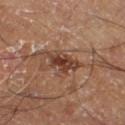Q: Was a biopsy performed?
A: catalogued during a skin exam; not biopsied
Q: Lesion location?
A: the right thigh
Q: What is the lesion's diameter?
A: ≈5 mm
Q: How was this image acquired?
A: 15 mm crop, total-body photography
Q: Illumination type?
A: cross-polarized illumination
Q: Patient demographics?
A: male, aged around 60
Q: Automated lesion metrics?
A: an area of roughly 7 mm², an eccentricity of roughly 0.9, and two-axis asymmetry of about 0.35; a within-lesion color-variation index near 3.5/10 and a peripheral color-asymmetry measure near 1; an automated nevus-likeness rating near 45 out of 100 and a lesion-detection confidence of about 90/100